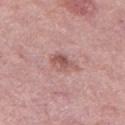biopsy status — imaged on a skin check; not biopsied
subject — female, aged approximately 40
location — the left thigh
acquisition — 15 mm crop, total-body photography